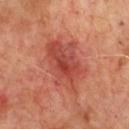Clinical impression:
No biopsy was performed on this lesion — it was imaged during a full skin examination and was not determined to be concerning.
Acquisition and patient details:
Imaged with cross-polarized lighting. About 7 mm across. Cropped from a whole-body photographic skin survey; the tile spans about 15 mm. The total-body-photography lesion software estimated a footprint of about 20 mm², a shape eccentricity near 0.8, and a symmetry-axis asymmetry near 0.25. And it measured a mean CIELAB color near L≈46 a*≈32 b*≈30 and roughly 10 lightness units darker than nearby skin. The analysis additionally found a border-irregularity index near 3.5/10, a color-variation rating of about 6/10, and radial color variation of about 2. On the chest. A male patient, in their 70s.